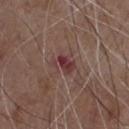Captured during whole-body skin photography for melanoma surveillance; the lesion was not biopsied.
A male patient aged approximately 80.
Located on the chest.
A lesion tile, about 15 mm wide, cut from a 3D total-body photograph.